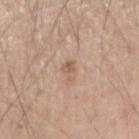This is a white-light tile. Cropped from a whole-body photographic skin survey; the tile spans about 15 mm. A male subject, in their 60s. The recorded lesion diameter is about 2.5 mm. The lesion is on the right forearm.